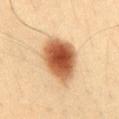<tbp_lesion>
  <site>abdomen</site>
  <image>
    <source>total-body photography crop</source>
    <field_of_view_mm>15</field_of_view_mm>
  </image>
  <patient>
    <sex>male</sex>
    <age_approx>35</age_approx>
  </patient>
  <lesion_size>
    <long_diameter_mm_approx>6.5</long_diameter_mm_approx>
  </lesion_size>
</tbp_lesion>